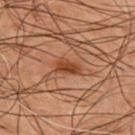- site: the back
- diameter: ~3.5 mm (longest diameter)
- subject: male, in their 50s
- image: 15 mm crop, total-body photography